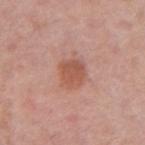{"biopsy_status": "not biopsied; imaged during a skin examination", "patient": {"sex": "female", "age_approx": 60}, "image": {"source": "total-body photography crop", "field_of_view_mm": 15}, "site": "arm"}